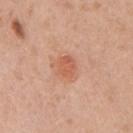This lesion was catalogued during total-body skin photography and was not selected for biopsy.
This is a white-light tile.
A close-up tile cropped from a whole-body skin photograph, about 15 mm across.
On the chest.
A male patient, about 55 years old.
Longest diameter approximately 2.5 mm.
Automated tile analysis of the lesion measured an area of roughly 4.5 mm², an eccentricity of roughly 0.6, and a shape-asymmetry score of about 0.15 (0 = symmetric). The software also gave a lesion color around L≈59 a*≈27 b*≈34 in CIELAB and roughly 8 lightness units darker than nearby skin. The analysis additionally found a detector confidence of about 100 out of 100 that the crop contains a lesion.